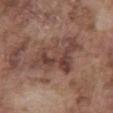Case summary:
- biopsy status · total-body-photography surveillance lesion; no biopsy
- automated metrics · about 9 CIELAB-L* units darker than the surrounding skin; a peripheral color-asymmetry measure near 2; a nevus-likeness score of about 0/100 and lesion-presence confidence of about 65/100
- subject · male, roughly 75 years of age
- illumination · white-light
- imaging modality · 15 mm crop, total-body photography
- anatomic site · the abdomen
- lesion diameter · ≈7.5 mm